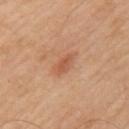Assessment: The lesion was tiled from a total-body skin photograph and was not biopsied. Image and clinical context: A male patient roughly 55 years of age. The lesion is located on the left upper arm. Imaged with cross-polarized lighting. This image is a 15 mm lesion crop taken from a total-body photograph. The total-body-photography lesion software estimated border irregularity of about 3 on a 0–10 scale, a color-variation rating of about 2/10, and radial color variation of about 0.5. The analysis additionally found lesion-presence confidence of about 100/100.